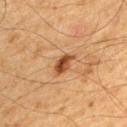<tbp_lesion>
  <biopsy_status>not biopsied; imaged during a skin examination</biopsy_status>
  <patient>
    <sex>male</sex>
    <age_approx>65</age_approx>
  </patient>
  <automated_metrics>
    <cielab_L>40</cielab_L>
    <cielab_a>21</cielab_a>
    <cielab_b>33</cielab_b>
    <vs_skin_contrast_norm>10.5</vs_skin_contrast_norm>
    <border_irregularity_0_10>3.0</border_irregularity_0_10>
    <color_variation_0_10>3.5</color_variation_0_10>
    <peripheral_color_asymmetry>1.5</peripheral_color_asymmetry>
    <nevus_likeness_0_100>95</nevus_likeness_0_100>
    <lesion_detection_confidence_0_100>100</lesion_detection_confidence_0_100>
  </automated_metrics>
  <site>back</site>
  <image>
    <source>total-body photography crop</source>
    <field_of_view_mm>15</field_of_view_mm>
  </image>
</tbp_lesion>